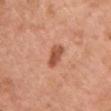Q: Is there a histopathology result?
A: total-body-photography surveillance lesion; no biopsy
Q: What kind of image is this?
A: 15 mm crop, total-body photography
Q: Where on the body is the lesion?
A: the front of the torso
Q: Lesion size?
A: ~3 mm (longest diameter)
Q: Illumination type?
A: white-light
Q: What are the patient's age and sex?
A: female, about 60 years old